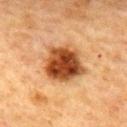Imaged during a routine full-body skin examination; the lesion was not biopsied and no histopathology is available.
The subject is a female approximately 60 years of age.
Imaged with cross-polarized lighting.
This image is a 15 mm lesion crop taken from a total-body photograph.
From the upper back.